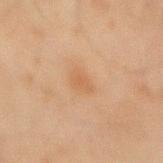Notes:
- notes: no biopsy performed (imaged during a skin exam)
- anatomic site: the mid back
- subject: male, approximately 45 years of age
- imaging modality: ~15 mm tile from a whole-body skin photo